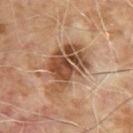<record>
<biopsy_status>not biopsied; imaged during a skin examination</biopsy_status>
<site>upper back</site>
<image>
  <source>total-body photography crop</source>
  <field_of_view_mm>15</field_of_view_mm>
</image>
<patient>
  <sex>male</sex>
  <age_approx>65</age_approx>
</patient>
<lesion_size>
  <long_diameter_mm_approx>6.5</long_diameter_mm_approx>
</lesion_size>
<lighting>cross-polarized</lighting>
</record>